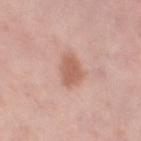Q: Was this lesion biopsied?
A: imaged on a skin check; not biopsied
Q: How large is the lesion?
A: about 3.5 mm
Q: How was the tile lit?
A: white-light illumination
Q: What kind of image is this?
A: 15 mm crop, total-body photography
Q: Where on the body is the lesion?
A: the left thigh
Q: Patient demographics?
A: female, aged 48–52